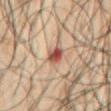The lesion was photographed on a routine skin check and not biopsied; there is no pathology result. The lesion is located on the abdomen. The total-body-photography lesion software estimated about 12 CIELAB-L* units darker than the surrounding skin and a normalized lesion–skin contrast near 10. The software also gave peripheral color asymmetry of about 2.5. Imaged with cross-polarized lighting. Approximately 2.5 mm at its widest. A male subject, approximately 50 years of age. Cropped from a total-body skin-imaging series; the visible field is about 15 mm.